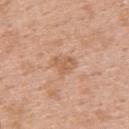Impression:
This lesion was catalogued during total-body skin photography and was not selected for biopsy.
Background:
The tile uses white-light illumination. A roughly 15 mm field-of-view crop from a total-body skin photograph. Automated image analysis of the tile measured a lesion area of about 3 mm², a shape eccentricity near 0.7, and a symmetry-axis asymmetry near 0.55. The software also gave roughly 8 lightness units darker than nearby skin and a normalized border contrast of about 5.5. The software also gave a border-irregularity index near 5.5/10, internal color variation of about 0 on a 0–10 scale, and peripheral color asymmetry of about 0. The software also gave a nevus-likeness score of about 0/100 and a lesion-detection confidence of about 100/100. A female patient aged 48–52. About 2.5 mm across. On the upper back.